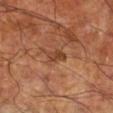Impression:
The lesion was photographed on a routine skin check and not biopsied; there is no pathology result.
Clinical summary:
A close-up tile cropped from a whole-body skin photograph, about 15 mm across. The patient is a male approximately 60 years of age. The lesion is on the right lower leg.